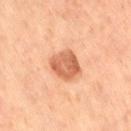The lesion was photographed on a routine skin check and not biopsied; there is no pathology result. A close-up tile cropped from a whole-body skin photograph, about 15 mm across. Located on the right thigh. The patient is a female aged around 65. Captured under cross-polarized illumination. The recorded lesion diameter is about 3.5 mm.